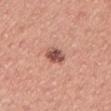Imaged with white-light lighting. The patient is a male roughly 40 years of age. A roughly 15 mm field-of-view crop from a total-body skin photograph. Located on the left upper arm. The lesion's longest dimension is about 2.5 mm. The lesion-visualizer software estimated an average lesion color of about L≈52 a*≈25 b*≈27 (CIELAB). The software also gave a nevus-likeness score of about 75/100 and a detector confidence of about 100 out of 100 that the crop contains a lesion.